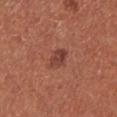The lesion was tiled from a total-body skin photograph and was not biopsied.
The lesion is located on the left lower leg.
A roughly 15 mm field-of-view crop from a total-body skin photograph.
A male subject, in their 70s.